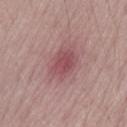Q: Lesion location?
A: the lower back
Q: What kind of image is this?
A: total-body-photography crop, ~15 mm field of view
Q: Patient demographics?
A: male, approximately 70 years of age